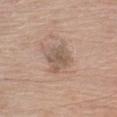No biopsy was performed on this lesion — it was imaged during a full skin examination and was not determined to be concerning.